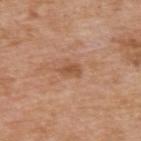Q: Was this lesion biopsied?
A: imaged on a skin check; not biopsied
Q: Who is the patient?
A: male, aged around 60
Q: Where on the body is the lesion?
A: the back
Q: How was this image acquired?
A: 15 mm crop, total-body photography
Q: What did automated image analysis measure?
A: about 9 CIELAB-L* units darker than the surrounding skin and a lesion-to-skin contrast of about 6.5 (normalized; higher = more distinct); a classifier nevus-likeness of about 0/100 and a lesion-detection confidence of about 100/100
Q: How was the tile lit?
A: white-light